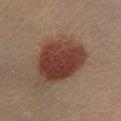follow-up=total-body-photography surveillance lesion; no biopsy
subject=male, roughly 40 years of age
lighting=cross-polarized
location=the left lower leg
imaging modality=15 mm crop, total-body photography